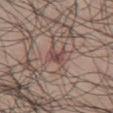Q: Is there a histopathology result?
A: no biopsy performed (imaged during a skin exam)
Q: What did automated image analysis measure?
A: a symmetry-axis asymmetry near 0.3; a within-lesion color-variation index near 3/10 and radial color variation of about 1; a classifier nevus-likeness of about 0/100 and lesion-presence confidence of about 75/100
Q: What are the patient's age and sex?
A: male, in their mid- to late 60s
Q: How was this image acquired?
A: ~15 mm tile from a whole-body skin photo
Q: Lesion location?
A: the leg
Q: Lesion size?
A: ≈2.5 mm